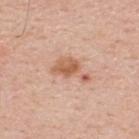{"biopsy_status": "not biopsied; imaged during a skin examination", "patient": {"sex": "male", "age_approx": 35}, "site": "upper back", "image": {"source": "total-body photography crop", "field_of_view_mm": 15}}